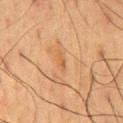The lesion is on the mid back.
A male subject about 60 years old.
A region of skin cropped from a whole-body photographic capture, roughly 15 mm wide.
Longest diameter approximately 2.5 mm.